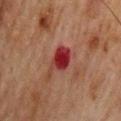This lesion was catalogued during total-body skin photography and was not selected for biopsy. This is a cross-polarized tile. This image is a 15 mm lesion crop taken from a total-body photograph. The lesion is on the mid back. The total-body-photography lesion software estimated a footprint of about 7 mm² and an eccentricity of roughly 0.85. The analysis additionally found a lesion color around L≈29 a*≈30 b*≈23 in CIELAB and a normalized border contrast of about 11.5. And it measured a border-irregularity rating of about 4/10, a color-variation rating of about 5/10, and radial color variation of about 1.5. The subject is a male aged 68–72. The lesion's longest dimension is about 4 mm.